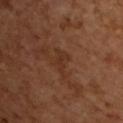Impression:
This lesion was catalogued during total-body skin photography and was not selected for biopsy.
Acquisition and patient details:
The tile uses cross-polarized illumination. The subject is a male aged 63 to 67. A 15 mm close-up tile from a total-body photography series done for melanoma screening. Measured at roughly 3.5 mm in maximum diameter.